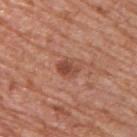biopsy status: imaged on a skin check; not biopsied | subject: male, in their mid- to late 60s | site: the upper back | illumination: white-light | imaging modality: 15 mm crop, total-body photography.